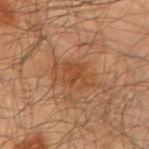Q: Is there a histopathology result?
A: no biopsy performed (imaged during a skin exam)
Q: Lesion location?
A: the right upper arm
Q: Patient demographics?
A: male, about 65 years old
Q: How was this image acquired?
A: total-body-photography crop, ~15 mm field of view